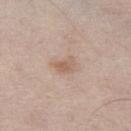Imaged during a routine full-body skin examination; the lesion was not biopsied and no histopathology is available.
A roughly 15 mm field-of-view crop from a total-body skin photograph.
The recorded lesion diameter is about 3 mm.
On the left thigh.
A male patient about 60 years old.
The tile uses white-light illumination.
The lesion-visualizer software estimated a lesion area of about 4 mm², an eccentricity of roughly 0.8, and two-axis asymmetry of about 0.45. It also reported border irregularity of about 4 on a 0–10 scale and radial color variation of about 0.5.